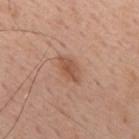Part of a total-body skin-imaging series; this lesion was reviewed on a skin check and was not flagged for biopsy. A male patient, roughly 60 years of age. Cropped from a total-body skin-imaging series; the visible field is about 15 mm. An algorithmic analysis of the crop reported an average lesion color of about L≈53 a*≈23 b*≈31 (CIELAB), a lesion–skin lightness drop of about 9, and a lesion-to-skin contrast of about 7 (normalized; higher = more distinct). About 3.5 mm across. Imaged with white-light lighting. From the upper back.